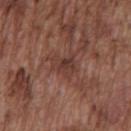The total-body-photography lesion software estimated a footprint of about 7 mm², an outline eccentricity of about 0.55 (0 = round, 1 = elongated), and two-axis asymmetry of about 0.4. The analysis additionally found an average lesion color of about L≈38 a*≈21 b*≈24 (CIELAB). The software also gave a border-irregularity rating of about 4.5/10, a color-variation rating of about 3/10, and peripheral color asymmetry of about 1.
A male subject about 75 years old.
The lesion is located on the front of the torso.
About 3.5 mm across.
Imaged with white-light lighting.
This image is a 15 mm lesion crop taken from a total-body photograph.